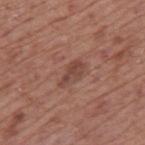Notes:
* follow-up · imaged on a skin check; not biopsied
* tile lighting · white-light illumination
* anatomic site · the mid back
* automated metrics · a mean CIELAB color near L≈44 a*≈22 b*≈25 and a normalized lesion–skin contrast near 6.5
* patient · male, in their mid-70s
* lesion diameter · ~3.5 mm (longest diameter)
* image · ~15 mm crop, total-body skin-cancer survey